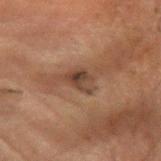workup: catalogued during a skin exam; not biopsied | imaging modality: ~15 mm crop, total-body skin-cancer survey | tile lighting: cross-polarized | anatomic site: the right forearm | size: ~3 mm (longest diameter) | subject: male, aged around 65.